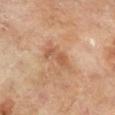workup: catalogued during a skin exam; not biopsied.